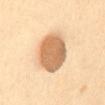follow-up = no biopsy performed (imaged during a skin exam)
acquisition = 15 mm crop, total-body photography
site = the abdomen
patient = female, in their 60s
lesion diameter = about 5.5 mm
lighting = cross-polarized illumination